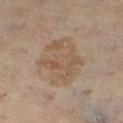Imaged during a routine full-body skin examination; the lesion was not biopsied and no histopathology is available.
A close-up tile cropped from a whole-body skin photograph, about 15 mm across.
Automated image analysis of the tile measured a footprint of about 24 mm² and two-axis asymmetry of about 0.2. The analysis additionally found an average lesion color of about L≈54 a*≈15 b*≈29 (CIELAB), about 7 CIELAB-L* units darker than the surrounding skin, and a lesion-to-skin contrast of about 5.5 (normalized; higher = more distinct). The software also gave a nevus-likeness score of about 10/100.
From the left lower leg.
The subject is a female aged 43 to 47.